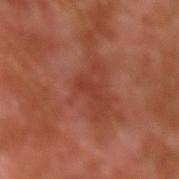Assessment: No biopsy was performed on this lesion — it was imaged during a full skin examination and was not determined to be concerning. Context: A male subject, in their 30s. On the left upper arm. Cropped from a total-body skin-imaging series; the visible field is about 15 mm.